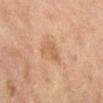<record>
<biopsy_status>not biopsied; imaged during a skin examination</biopsy_status>
<image>
  <source>total-body photography crop</source>
  <field_of_view_mm>15</field_of_view_mm>
</image>
<lighting>cross-polarized</lighting>
<lesion_size>
  <long_diameter_mm_approx>2.5</long_diameter_mm_approx>
</lesion_size>
<site>leg</site>
<automated_metrics>
  <area_mm2_approx>4.5</area_mm2_approx>
  <shape_asymmetry>0.45</shape_asymmetry>
</automated_metrics>
<patient>
  <sex>female</sex>
  <age_approx>55</age_approx>
</patient>
</record>